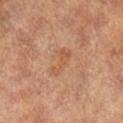Q: Was this lesion biopsied?
A: catalogued during a skin exam; not biopsied
Q: Who is the patient?
A: female, aged around 75
Q: What did automated image analysis measure?
A: a footprint of about 4.5 mm² and a shape-asymmetry score of about 0.4 (0 = symmetric)
Q: How was the tile lit?
A: cross-polarized
Q: How was this image acquired?
A: ~15 mm crop, total-body skin-cancer survey
Q: Where on the body is the lesion?
A: the left lower leg